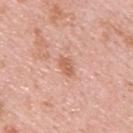<case>
  <biopsy_status>not biopsied; imaged during a skin examination</biopsy_status>
  <patient>
    <sex>male</sex>
    <age_approx>25</age_approx>
  </patient>
  <site>upper back</site>
  <image>
    <source>total-body photography crop</source>
    <field_of_view_mm>15</field_of_view_mm>
  </image>
</case>